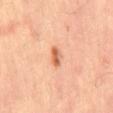follow-up: total-body-photography surveillance lesion; no biopsy
body site: the mid back
subject: male, aged approximately 65
acquisition: 15 mm crop, total-body photography
lesion diameter: about 3 mm
TBP lesion metrics: a mean CIELAB color near L≈63 a*≈27 b*≈37 and roughly 12 lightness units darker than nearby skin; border irregularity of about 2 on a 0–10 scale, internal color variation of about 4.5 on a 0–10 scale, and peripheral color asymmetry of about 1.5; an automated nevus-likeness rating near 90 out of 100 and lesion-presence confidence of about 100/100